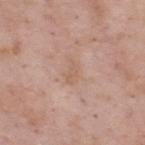{"biopsy_status": "not biopsied; imaged during a skin examination", "image": {"source": "total-body photography crop", "field_of_view_mm": 15}, "patient": {"sex": "male", "age_approx": 55}, "site": "upper back", "lighting": "white-light", "lesion_size": {"long_diameter_mm_approx": 3.0}}